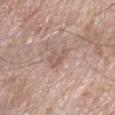{"biopsy_status": "not biopsied; imaged during a skin examination", "lighting": "white-light", "image": {"source": "total-body photography crop", "field_of_view_mm": 15}, "site": "left forearm", "patient": {"sex": "male", "age_approx": 60}, "automated_metrics": {"area_mm2_approx": 4.0, "eccentricity": 0.7, "shape_asymmetry": 0.4, "cielab_L": 57, "cielab_a": 17, "cielab_b": 25, "vs_skin_darker_L": 7.0, "vs_skin_contrast_norm": 5.0, "nevus_likeness_0_100": 0, "lesion_detection_confidence_0_100": 100}, "lesion_size": {"long_diameter_mm_approx": 3.0}}